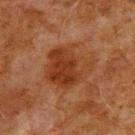Findings:
• workup · total-body-photography surveillance lesion; no biopsy
• diameter · ≈6 mm
• body site · the back
• tile lighting · cross-polarized illumination
• subject · male, aged approximately 80
• imaging modality · ~15 mm tile from a whole-body skin photo
• image-analysis metrics · a footprint of about 22 mm²; a mean CIELAB color near L≈30 a*≈21 b*≈29, a lesion–skin lightness drop of about 7, and a lesion-to-skin contrast of about 8 (normalized; higher = more distinct); a nevus-likeness score of about 5/100 and a lesion-detection confidence of about 100/100